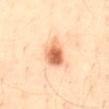No biopsy was performed on this lesion — it was imaged during a full skin examination and was not determined to be concerning. Located on the abdomen. The subject is a male in their 40s. A 15 mm close-up tile from a total-body photography series done for melanoma screening. The lesion's longest dimension is about 4 mm.